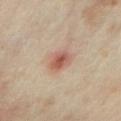| key | value |
|---|---|
| workup | imaged on a skin check; not biopsied |
| illumination | cross-polarized |
| image source | total-body-photography crop, ~15 mm field of view |
| subject | female, aged 33 to 37 |
| location | the arm |
| lesion diameter | ≈2.5 mm |